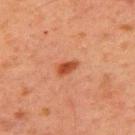lesion size = ~2.5 mm (longest diameter); subject = male, about 60 years old; image source = ~15 mm crop, total-body skin-cancer survey; anatomic site = the upper back; tile lighting = cross-polarized illumination.